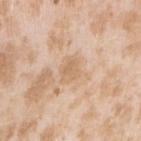Clinical impression:
Imaged during a routine full-body skin examination; the lesion was not biopsied and no histopathology is available.
Image and clinical context:
A female patient, in their mid- to late 20s. A close-up tile cropped from a whole-body skin photograph, about 15 mm across. The lesion is located on the right upper arm.